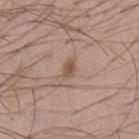Notes:
– biopsy status — total-body-photography surveillance lesion; no biopsy
– image-analysis metrics — a lesion area of about 3 mm² and two-axis asymmetry of about 0.3
– illumination — white-light
– site — the chest
– acquisition — 15 mm crop, total-body photography
– patient — male, in their mid- to late 50s
– lesion diameter — ≈2.5 mm